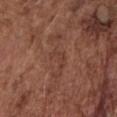Imaged during a routine full-body skin examination; the lesion was not biopsied and no histopathology is available. A lesion tile, about 15 mm wide, cut from a 3D total-body photograph. Located on the front of the torso. The subject is a male aged around 75.